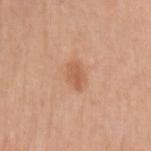Clinical impression: The lesion was tiled from a total-body skin photograph and was not biopsied. Clinical summary: Approximately 2.5 mm at its widest. Automated tile analysis of the lesion measured about 9 CIELAB-L* units darker than the surrounding skin and a normalized border contrast of about 6.5. The patient is a female aged approximately 50. This is a white-light tile. The lesion is located on the left upper arm. A 15 mm close-up tile from a total-body photography series done for melanoma screening.